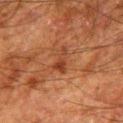notes = total-body-photography surveillance lesion; no biopsy | image source = 15 mm crop, total-body photography | anatomic site = the right thigh | lighting = cross-polarized | patient = male, approximately 80 years of age.